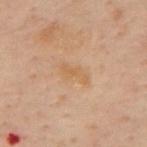Impression: Part of a total-body skin-imaging series; this lesion was reviewed on a skin check and was not flagged for biopsy. Image and clinical context: A roughly 15 mm field-of-view crop from a total-body skin photograph. Imaged with cross-polarized lighting. An algorithmic analysis of the crop reported an average lesion color of about L≈49 a*≈15 b*≈31 (CIELAB), roughly 5 lightness units darker than nearby skin, and a normalized lesion–skin contrast near 5.5. The software also gave a border-irregularity index near 4.5/10, a within-lesion color-variation index near 1.5/10, and a peripheral color-asymmetry measure near 0.5. A male subject roughly 50 years of age. The lesion's longest dimension is about 3.5 mm. Located on the mid back.